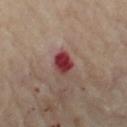Clinical impression:
Captured during whole-body skin photography for melanoma surveillance; the lesion was not biopsied.
Background:
The lesion's longest dimension is about 3 mm. A 15 mm close-up extracted from a 3D total-body photography capture. The total-body-photography lesion software estimated an area of roughly 5 mm², an eccentricity of roughly 0.7, and two-axis asymmetry of about 0.15. The software also gave a mean CIELAB color near L≈37 a*≈31 b*≈21, roughly 16 lightness units darker than nearby skin, and a normalized border contrast of about 13. The analysis additionally found a nevus-likeness score of about 0/100. A female patient, aged 58–62. On the left thigh.